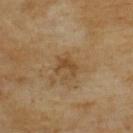Findings:
• workup: catalogued during a skin exam; not biopsied
• patient: female, about 60 years old
• anatomic site: the upper back
• image: ~15 mm tile from a whole-body skin photo